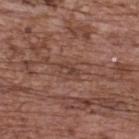The lesion was tiled from a total-body skin photograph and was not biopsied. A region of skin cropped from a whole-body photographic capture, roughly 15 mm wide. The total-body-photography lesion software estimated a footprint of about 2.5 mm² and a shape-asymmetry score of about 0.2 (0 = symmetric). It also reported a lesion color around L≈41 a*≈20 b*≈24 in CIELAB and a normalized border contrast of about 6. It also reported an automated nevus-likeness rating near 0 out of 100 and lesion-presence confidence of about 80/100. From the upper back. The subject is a female about 65 years old.